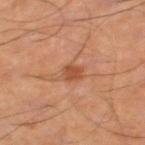subject: male, approximately 55 years of age; location: the leg; tile lighting: cross-polarized illumination; automated metrics: a classifier nevus-likeness of about 60/100; lesion diameter: about 2 mm; image: total-body-photography crop, ~15 mm field of view.